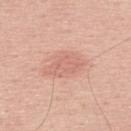| feature | finding |
|---|---|
| follow-up | total-body-photography surveillance lesion; no biopsy |
| imaging modality | ~15 mm crop, total-body skin-cancer survey |
| patient | male, roughly 65 years of age |
| location | the upper back |
| automated lesion analysis | a shape eccentricity near 0.8 and two-axis asymmetry of about 0.25; a mean CIELAB color near L≈66 a*≈24 b*≈28 and a normalized lesion–skin contrast near 5; an automated nevus-likeness rating near 25 out of 100 |
| lesion diameter | about 5 mm |
| lighting | white-light illumination |